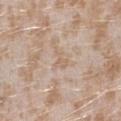Clinical impression:
Captured during whole-body skin photography for melanoma surveillance; the lesion was not biopsied.
Acquisition and patient details:
A lesion tile, about 15 mm wide, cut from a 3D total-body photograph. Captured under white-light illumination. About 2.5 mm across. The patient is a female aged approximately 25. From the left forearm. The lesion-visualizer software estimated a lesion color around L≈63 a*≈15 b*≈29 in CIELAB, a lesion–skin lightness drop of about 6, and a normalized lesion–skin contrast near 4.5. And it measured a border-irregularity index near 5.5/10, internal color variation of about 0 on a 0–10 scale, and a peripheral color-asymmetry measure near 0.